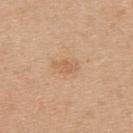<tbp_lesion>
<image>
  <source>total-body photography crop</source>
  <field_of_view_mm>15</field_of_view_mm>
</image>
<site>upper back</site>
<lesion_size>
  <long_diameter_mm_approx>3.0</long_diameter_mm_approx>
</lesion_size>
<lighting>white-light</lighting>
<patient>
  <sex>female</sex>
  <age_approx>40</age_approx>
</patient>
</tbp_lesion>